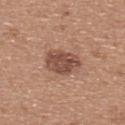Impression: No biopsy was performed on this lesion — it was imaged during a full skin examination and was not determined to be concerning. Background: A 15 mm close-up extracted from a 3D total-body photography capture. On the upper back. A female patient, aged around 35. This is a white-light tile.